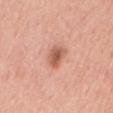Recorded during total-body skin imaging; not selected for excision or biopsy.
On the mid back.
A 15 mm crop from a total-body photograph taken for skin-cancer surveillance.
A male patient, roughly 60 years of age.
Imaged with white-light lighting.
Longest diameter approximately 3 mm.